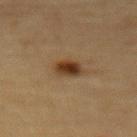Assessment:
Recorded during total-body skin imaging; not selected for excision or biopsy.
Context:
Measured at roughly 2.5 mm in maximum diameter. Automated image analysis of the tile measured an eccentricity of roughly 0.5 and a shape-asymmetry score of about 0.2 (0 = symmetric). It also reported a nevus-likeness score of about 100/100. A region of skin cropped from a whole-body photographic capture, roughly 15 mm wide. A male subject in their mid- to late 80s or older. On the abdomen. Captured under cross-polarized illumination.